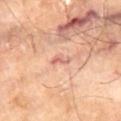Assessment:
No biopsy was performed on this lesion — it was imaged during a full skin examination and was not determined to be concerning.
Image and clinical context:
The lesion-visualizer software estimated a mean CIELAB color near L≈64 a*≈25 b*≈29, a lesion–skin lightness drop of about 8, and a normalized border contrast of about 5.5. The analysis additionally found an automated nevus-likeness rating near 0 out of 100. A region of skin cropped from a whole-body photographic capture, roughly 15 mm wide. This is a cross-polarized tile. Measured at roughly 3 mm in maximum diameter. On the leg. A male patient aged approximately 70.